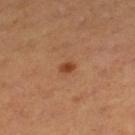• follow-up · total-body-photography surveillance lesion; no biopsy
• illumination · cross-polarized illumination
• anatomic site · the leg
• lesion size · ~2 mm (longest diameter)
• patient · female, aged 28–32
• image source · ~15 mm crop, total-body skin-cancer survey